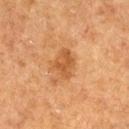| key | value |
|---|---|
| workup | catalogued during a skin exam; not biopsied |
| image | ~15 mm crop, total-body skin-cancer survey |
| site | the leg |
| tile lighting | cross-polarized illumination |
| patient | male, aged 73–77 |
| diameter | ≈3.5 mm |